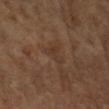- patient: male, aged approximately 60
- location: the left forearm
- image source: ~15 mm crop, total-body skin-cancer survey